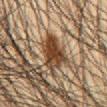Assessment: Recorded during total-body skin imaging; not selected for excision or biopsy. Context: The lesion is located on the abdomen. A male subject, aged approximately 45. Cropped from a whole-body photographic skin survey; the tile spans about 15 mm.